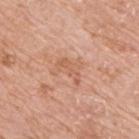Q: Was a biopsy performed?
A: imaged on a skin check; not biopsied
Q: Where on the body is the lesion?
A: the back
Q: Patient demographics?
A: male, in their mid-70s
Q: What is the imaging modality?
A: 15 mm crop, total-body photography